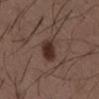Q: Was this lesion biopsied?
A: imaged on a skin check; not biopsied
Q: Automated lesion metrics?
A: a border-irregularity rating of about 2.5/10, a color-variation rating of about 3.5/10, and a peripheral color-asymmetry measure near 1; a detector confidence of about 100 out of 100 that the crop contains a lesion
Q: Where on the body is the lesion?
A: the front of the torso
Q: Illumination type?
A: white-light
Q: Lesion size?
A: ≈4 mm
Q: How was this image acquired?
A: total-body-photography crop, ~15 mm field of view
Q: Patient demographics?
A: male, aged 48–52